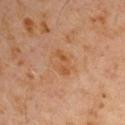The lesion was tiled from a total-body skin photograph and was not biopsied. A male subject about 60 years old. Imaged with cross-polarized lighting. The total-body-photography lesion software estimated about 7 CIELAB-L* units darker than the surrounding skin and a lesion-to-skin contrast of about 6.5 (normalized; higher = more distinct). The software also gave a border-irregularity rating of about 5/10, internal color variation of about 0.5 on a 0–10 scale, and radial color variation of about 0. On the arm. Cropped from a whole-body photographic skin survey; the tile spans about 15 mm.